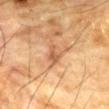follow-up — no biopsy performed (imaged during a skin exam) | location — the chest | illumination — cross-polarized | patient — male, in their mid- to late 80s or older | size — ≈2.5 mm | image — ~15 mm tile from a whole-body skin photo | automated lesion analysis — an area of roughly 3 mm², an eccentricity of roughly 0.8, and a shape-asymmetry score of about 0.35 (0 = symmetric); a lesion–skin lightness drop of about 9 and a normalized border contrast of about 6.5.